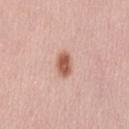  biopsy_status: not biopsied; imaged during a skin examination
  image:
    source: total-body photography crop
    field_of_view_mm: 15
  site: lower back
  patient:
    sex: female
    age_approx: 60